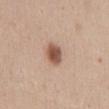Findings:
– follow-up · catalogued during a skin exam; not biopsied
– patient · female, aged approximately 30
– lighting · white-light
– image source · total-body-photography crop, ~15 mm field of view
– site · the chest